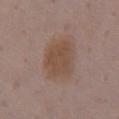Impression:
The lesion was photographed on a routine skin check and not biopsied; there is no pathology result.
Background:
A 15 mm close-up extracted from a 3D total-body photography capture. Located on the front of the torso. A female subject, in their 50s.